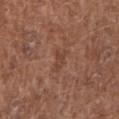biopsy status: no biopsy performed (imaged during a skin exam) | subject: female, aged around 50 | anatomic site: the right upper arm | imaging modality: ~15 mm crop, total-body skin-cancer survey.